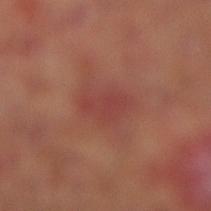Q: Is there a histopathology result?
A: no biopsy performed (imaged during a skin exam)
Q: What lighting was used for the tile?
A: cross-polarized
Q: What are the patient's age and sex?
A: male, about 65 years old
Q: What kind of image is this?
A: 15 mm crop, total-body photography
Q: What is the lesion's diameter?
A: about 4 mm
Q: What is the anatomic site?
A: the right lower leg